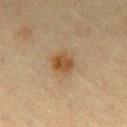Q: Is there a histopathology result?
A: no biopsy performed (imaged during a skin exam)
Q: How was this image acquired?
A: total-body-photography crop, ~15 mm field of view
Q: What is the anatomic site?
A: the mid back
Q: Who is the patient?
A: male, in their 70s